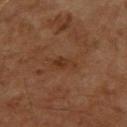Q: Was this lesion biopsied?
A: catalogued during a skin exam; not biopsied